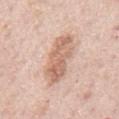Recorded during total-body skin imaging; not selected for excision or biopsy. A male subject aged 73 to 77. A 15 mm close-up extracted from a 3D total-body photography capture. This is a white-light tile. The lesion is located on the mid back. Measured at roughly 7.5 mm in maximum diameter.